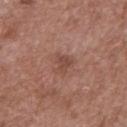Impression: No biopsy was performed on this lesion — it was imaged during a full skin examination and was not determined to be concerning. Clinical summary: Automated image analysis of the tile measured a symmetry-axis asymmetry near 0.45. The software also gave a mean CIELAB color near L≈47 a*≈21 b*≈26, a lesion–skin lightness drop of about 8, and a normalized lesion–skin contrast near 6. And it measured a border-irregularity rating of about 4/10, a within-lesion color-variation index near 2/10, and radial color variation of about 0.5. From the mid back. About 2.5 mm across. A close-up tile cropped from a whole-body skin photograph, about 15 mm across. The patient is a male in their 50s. Captured under white-light illumination.